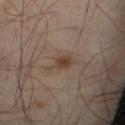follow-up = total-body-photography surveillance lesion; no biopsy | image source = ~15 mm crop, total-body skin-cancer survey | site = the abdomen | patient = male, roughly 50 years of age.